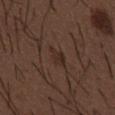Recorded during total-body skin imaging; not selected for excision or biopsy.
The tile uses white-light illumination.
This image is a 15 mm lesion crop taken from a total-body photograph.
The lesion is on the abdomen.
The subject is a male aged around 50.
The lesion's longest dimension is about 2.5 mm.